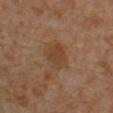Findings:
* follow-up: catalogued during a skin exam; not biopsied
* patient: male, aged 28 to 32
* automated lesion analysis: an automated nevus-likeness rating near 0 out of 100 and a detector confidence of about 100 out of 100 that the crop contains a lesion
* location: the left leg
* lesion diameter: ~3.5 mm (longest diameter)
* lighting: cross-polarized illumination
* imaging modality: ~15 mm tile from a whole-body skin photo